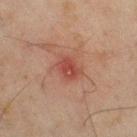notes: catalogued during a skin exam; not biopsied
lesion size: about 3 mm
patient: male, about 45 years old
automated lesion analysis: an average lesion color of about L≈38 a*≈24 b*≈25 (CIELAB), about 8 CIELAB-L* units darker than the surrounding skin, and a normalized border contrast of about 6.5; a border-irregularity rating of about 2.5/10, a color-variation rating of about 3/10, and peripheral color asymmetry of about 1
acquisition: ~15 mm tile from a whole-body skin photo
illumination: cross-polarized
site: the leg